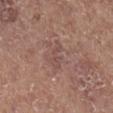Imaged during a routine full-body skin examination; the lesion was not biopsied and no histopathology is available. A male patient aged approximately 75. Located on the right lower leg. Automated tile analysis of the lesion measured a lesion area of about 4.5 mm², a shape eccentricity near 0.85, and two-axis asymmetry of about 0.4. The software also gave border irregularity of about 5 on a 0–10 scale, a color-variation rating of about 1.5/10, and radial color variation of about 0.5. Imaged with white-light lighting. Longest diameter approximately 3 mm. A 15 mm crop from a total-body photograph taken for skin-cancer surveillance.